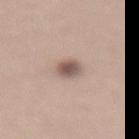Notes:
• lesion size: ≈3 mm
• patient: female, aged 28–32
• imaging modality: ~15 mm tile from a whole-body skin photo
• location: the lower back
• illumination: white-light illumination
• automated lesion analysis: an eccentricity of roughly 0.75 and a symmetry-axis asymmetry near 0.2; a mean CIELAB color near L≈56 a*≈16 b*≈22, a lesion–skin lightness drop of about 13, and a normalized border contrast of about 8.5